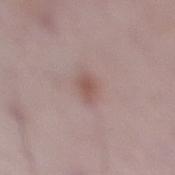• follow-up · imaged on a skin check; not biopsied
• location · the right lower leg
• patient · male, roughly 50 years of age
• diameter · ~2.5 mm (longest diameter)
• imaging modality · 15 mm crop, total-body photography
• lighting · white-light